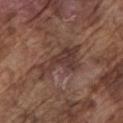Clinical summary:
Located on the arm. A male subject aged 73 to 77. The lesion's longest dimension is about 6.5 mm. Cropped from a total-body skin-imaging series; the visible field is about 15 mm.